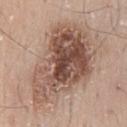notes = total-body-photography surveillance lesion; no biopsy | size = ~10.5 mm (longest diameter) | subject = male, aged 48 to 52 | anatomic site = the lower back | image = 15 mm crop, total-body photography | illumination = white-light illumination.